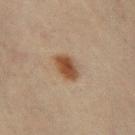workup = no biopsy performed (imaged during a skin exam) | patient = female, aged approximately 45 | image source = ~15 mm tile from a whole-body skin photo | lighting = cross-polarized | anatomic site = the left thigh.